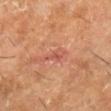This lesion was catalogued during total-body skin photography and was not selected for biopsy.
A male subject aged around 60.
Cropped from a whole-body photographic skin survey; the tile spans about 15 mm.
From the right lower leg.